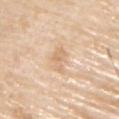Assessment:
This lesion was catalogued during total-body skin photography and was not selected for biopsy.
Context:
A roughly 15 mm field-of-view crop from a total-body skin photograph. A male subject, aged 78–82. This is a white-light tile. About 3.5 mm across. Automated tile analysis of the lesion measured an area of roughly 4.5 mm², a shape eccentricity near 0.8, and two-axis asymmetry of about 0.4. The software also gave an average lesion color of about L≈70 a*≈17 b*≈35 (CIELAB) and a normalized lesion–skin contrast near 5. And it measured a border-irregularity index near 4/10, a within-lesion color-variation index near 2/10, and a peripheral color-asymmetry measure near 0.5. It also reported a nevus-likeness score of about 0/100 and lesion-presence confidence of about 100/100. From the upper back.